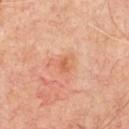Assessment:
The lesion was tiled from a total-body skin photograph and was not biopsied.
Background:
A lesion tile, about 15 mm wide, cut from a 3D total-body photograph. This is a cross-polarized tile. A male subject, roughly 65 years of age. About 2.5 mm across. Located on the chest.